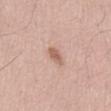The subject is a male in their mid- to late 50s. The lesion-visualizer software estimated an eccentricity of roughly 0.75 and a shape-asymmetry score of about 0.2 (0 = symmetric). The software also gave an automated nevus-likeness rating near 60 out of 100. Cropped from a total-body skin-imaging series; the visible field is about 15 mm. About 2.5 mm across. On the abdomen. Imaged with white-light lighting.